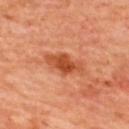patient = male, aged 58–62
imaging modality = ~15 mm tile from a whole-body skin photo
location = the back